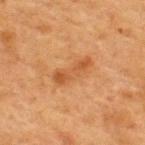Impression:
This lesion was catalogued during total-body skin photography and was not selected for biopsy.
Context:
A 15 mm crop from a total-body photograph taken for skin-cancer surveillance. Automated image analysis of the tile measured an automated nevus-likeness rating near 10 out of 100 and lesion-presence confidence of about 100/100. The patient is a female aged approximately 40. Located on the upper back.